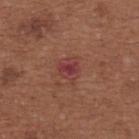notes = total-body-photography surveillance lesion; no biopsy | lesion size = ≈3 mm | automated lesion analysis = about 7 CIELAB-L* units darker than the surrounding skin and a normalized lesion–skin contrast near 7.5; a border-irregularity rating of about 2.5/10 and a color-variation rating of about 4/10; a classifier nevus-likeness of about 0/100 and a lesion-detection confidence of about 100/100 | image source = 15 mm crop, total-body photography | body site = the upper back | patient = female, aged 63 to 67.